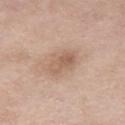Recorded during total-body skin imaging; not selected for excision or biopsy.
Located on the right thigh.
The subject is a female in their mid- to late 50s.
The tile uses white-light illumination.
The total-body-photography lesion software estimated a border-irregularity index near 2.5/10.
Measured at roughly 3.5 mm in maximum diameter.
A close-up tile cropped from a whole-body skin photograph, about 15 mm across.